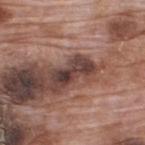Part of a total-body skin-imaging series; this lesion was reviewed on a skin check and was not flagged for biopsy. A lesion tile, about 15 mm wide, cut from a 3D total-body photograph. A male patient, aged 68–72. On the upper back. This is a white-light tile.